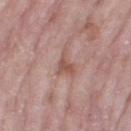{"biopsy_status": "not biopsied; imaged during a skin examination", "lighting": "white-light", "site": "right thigh", "image": {"source": "total-body photography crop", "field_of_view_mm": 15}, "automated_metrics": {"eccentricity": 0.8, "shape_asymmetry": 0.6, "border_irregularity_0_10": 6.0}, "lesion_size": {"long_diameter_mm_approx": 3.5}, "patient": {"sex": "female", "age_approx": 70}}